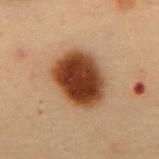Assessment: The lesion was photographed on a routine skin check and not biopsied; there is no pathology result. Context: Imaged with cross-polarized lighting. A male subject approximately 55 years of age. A close-up tile cropped from a whole-body skin photograph, about 15 mm across. Located on the mid back. Approximately 6 mm at its widest.